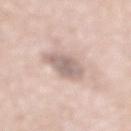This lesion was catalogued during total-body skin photography and was not selected for biopsy.
On the mid back.
An algorithmic analysis of the crop reported a mean CIELAB color near L≈64 a*≈15 b*≈21 and roughly 11 lightness units darker than nearby skin.
This is a white-light tile.
About 3.5 mm across.
A region of skin cropped from a whole-body photographic capture, roughly 15 mm wide.
A male patient approximately 80 years of age.